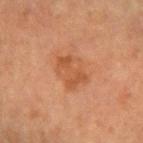The lesion was tiled from a total-body skin photograph and was not biopsied. A female subject, aged 53–57. Cropped from a whole-body photographic skin survey; the tile spans about 15 mm. Located on the arm. Automated tile analysis of the lesion measured a lesion area of about 9.5 mm², an outline eccentricity of about 0.7 (0 = round, 1 = elongated), and a shape-asymmetry score of about 0.25 (0 = symmetric). It also reported a lesion color around L≈46 a*≈23 b*≈34 in CIELAB and a normalized border contrast of about 6. It also reported peripheral color asymmetry of about 1. The software also gave a classifier nevus-likeness of about 5/100 and a lesion-detection confidence of about 100/100.